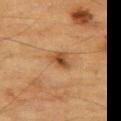| feature | finding |
|---|---|
| workup | catalogued during a skin exam; not biopsied |
| image | 15 mm crop, total-body photography |
| TBP lesion metrics | a mean CIELAB color near L≈43 a*≈19 b*≈34, roughly 10 lightness units darker than nearby skin, and a normalized border contrast of about 8; a nevus-likeness score of about 65/100 and lesion-presence confidence of about 100/100 |
| lesion diameter | ~3 mm (longest diameter) |
| tile lighting | cross-polarized |
| patient | male, approximately 85 years of age or older |
| anatomic site | the leg |